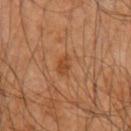Assessment: The lesion was tiled from a total-body skin photograph and was not biopsied. Context: The lesion is on the left upper arm. About 3.5 mm across. This image is a 15 mm lesion crop taken from a total-body photograph. A male subject, about 60 years old. The tile uses cross-polarized illumination. The total-body-photography lesion software estimated an outline eccentricity of about 0.6 (0 = round, 1 = elongated) and two-axis asymmetry of about 0.3. The software also gave internal color variation of about 4.5 on a 0–10 scale. The analysis additionally found lesion-presence confidence of about 100/100.